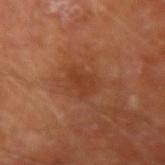Case summary:
* notes — no biopsy performed (imaged during a skin exam)
* site — the right forearm
* automated metrics — a shape-asymmetry score of about 0.4 (0 = symmetric)
* acquisition — ~15 mm crop, total-body skin-cancer survey
* tile lighting — cross-polarized illumination
* patient — male, aged around 65